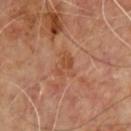Recorded during total-body skin imaging; not selected for excision or biopsy.